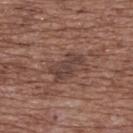Captured during whole-body skin photography for melanoma surveillance; the lesion was not biopsied.
A close-up tile cropped from a whole-body skin photograph, about 15 mm across.
About 4.5 mm across.
This is a white-light tile.
A female subject, in their mid-70s.
From the upper back.